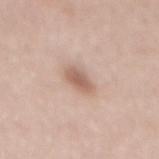* biopsy status — total-body-photography surveillance lesion; no biopsy
* patient — female, about 60 years old
* tile lighting — white-light illumination
* image source — total-body-photography crop, ~15 mm field of view
* location — the back